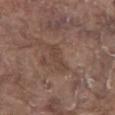Imaged during a routine full-body skin examination; the lesion was not biopsied and no histopathology is available. On the chest. A 15 mm crop from a total-body photograph taken for skin-cancer surveillance. A male patient roughly 80 years of age. Automated image analysis of the tile measured an outline eccentricity of about 0.95 (0 = round, 1 = elongated). The analysis additionally found a lesion color around L≈42 a*≈17 b*≈24 in CIELAB and a normalized border contrast of about 5. The analysis additionally found an automated nevus-likeness rating near 0 out of 100 and a detector confidence of about 65 out of 100 that the crop contains a lesion. The tile uses white-light illumination. The recorded lesion diameter is about 3.5 mm.